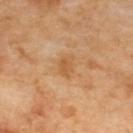This lesion was catalogued during total-body skin photography and was not selected for biopsy.
Approximately 2.5 mm at its widest.
Captured under cross-polarized illumination.
Cropped from a total-body skin-imaging series; the visible field is about 15 mm.
The lesion-visualizer software estimated an average lesion color of about L≈58 a*≈22 b*≈42 (CIELAB) and roughly 8 lightness units darker than nearby skin. The analysis additionally found a border-irregularity index near 2.5/10, a color-variation rating of about 2/10, and a peripheral color-asymmetry measure near 0.5. And it measured an automated nevus-likeness rating near 0 out of 100.
From the upper back.